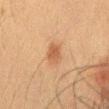  lesion_size:
    long_diameter_mm_approx: 3.0
  lighting: cross-polarized
  site: mid back
  patient:
    sex: male
    age_approx: 35
  image:
    source: total-body photography crop
    field_of_view_mm: 15
  automated_metrics:
    area_mm2_approx: 5.5
    eccentricity: 0.75
    shape_asymmetry: 0.2
    cielab_L: 45
    cielab_a: 19
    cielab_b: 30
    vs_skin_darker_L: 8.0
    vs_skin_contrast_norm: 6.0
    nevus_likeness_0_100: 80
    lesion_detection_confidence_0_100: 100